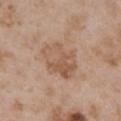Notes:
- notes · no biopsy performed (imaged during a skin exam)
- patient · female, about 30 years old
- site · the upper back
- lesion diameter · about 5 mm
- imaging modality · ~15 mm tile from a whole-body skin photo
- automated lesion analysis · roughly 8 lightness units darker than nearby skin and a lesion-to-skin contrast of about 6 (normalized; higher = more distinct); a nevus-likeness score of about 0/100 and a detector confidence of about 100 out of 100 that the crop contains a lesion